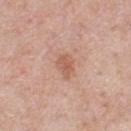biopsy status: total-body-photography surveillance lesion; no biopsy
site: the chest
lesion diameter: ≈3 mm
image: 15 mm crop, total-body photography
subject: male, aged 38 to 42